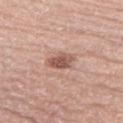{"biopsy_status": "not biopsied; imaged during a skin examination", "lighting": "white-light", "image": {"source": "total-body photography crop", "field_of_view_mm": 15}, "lesion_size": {"long_diameter_mm_approx": 3.5}, "patient": {"sex": "male", "age_approx": 70}, "site": "left lower leg"}